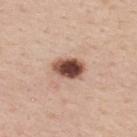Part of a total-body skin-imaging series; this lesion was reviewed on a skin check and was not flagged for biopsy. Cropped from a whole-body photographic skin survey; the tile spans about 15 mm. A female subject, roughly 55 years of age. About 4 mm across. Located on the upper back.